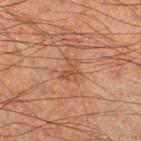follow-up=total-body-photography surveillance lesion; no biopsy | patient=male, in their mid- to late 60s | image=total-body-photography crop, ~15 mm field of view | site=the right lower leg | illumination=cross-polarized | lesion size=about 2.5 mm.